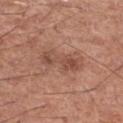The lesion was tiled from a total-body skin photograph and was not biopsied.
Captured under white-light illumination.
A 15 mm close-up extracted from a 3D total-body photography capture.
On the left thigh.
Automated image analysis of the tile measured an area of roughly 9.5 mm², an outline eccentricity of about 0.9 (0 = round, 1 = elongated), and two-axis asymmetry of about 0.3. The analysis additionally found a nevus-likeness score of about 20/100 and lesion-presence confidence of about 100/100.
The subject is a male aged around 65.
Measured at roughly 5 mm in maximum diameter.